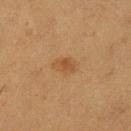Impression:
This lesion was catalogued during total-body skin photography and was not selected for biopsy.
Context:
A region of skin cropped from a whole-body photographic capture, roughly 15 mm wide. This is a cross-polarized tile. A female patient aged 38–42. The lesion's longest dimension is about 3 mm. On the left lower leg.